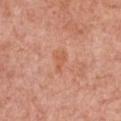Clinical impression: No biopsy was performed on this lesion — it was imaged during a full skin examination and was not determined to be concerning. Clinical summary: The lesion is located on the chest. This is a white-light tile. A female subject about 60 years old. Approximately 2.5 mm at its widest. Automated tile analysis of the lesion measured an average lesion color of about L≈59 a*≈27 b*≈35 (CIELAB), about 6 CIELAB-L* units darker than the surrounding skin, and a lesion-to-skin contrast of about 5 (normalized; higher = more distinct). It also reported a border-irregularity rating of about 4/10, a color-variation rating of about 0.5/10, and peripheral color asymmetry of about 0. The software also gave a nevus-likeness score of about 0/100 and a detector confidence of about 100 out of 100 that the crop contains a lesion. A close-up tile cropped from a whole-body skin photograph, about 15 mm across.